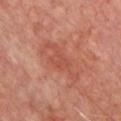Q: Was a biopsy performed?
A: no biopsy performed (imaged during a skin exam)